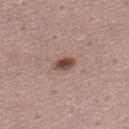Notes:
- biopsy status · imaged on a skin check; not biopsied